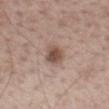No biopsy was performed on this lesion — it was imaged during a full skin examination and was not determined to be concerning.
A male patient, aged 58–62.
On the mid back.
A close-up tile cropped from a whole-body skin photograph, about 15 mm across.